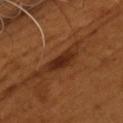{
  "biopsy_status": "not biopsied; imaged during a skin examination",
  "site": "chest",
  "patient": {
    "sex": "male",
    "age_approx": 55
  },
  "image": {
    "source": "total-body photography crop",
    "field_of_view_mm": 15
  },
  "lesion_size": {
    "long_diameter_mm_approx": 3.5
  },
  "automated_metrics": {
    "vs_skin_darker_L": 10.0,
    "vs_skin_contrast_norm": 10.0,
    "border_irregularity_0_10": 3.5,
    "color_variation_0_10": 2.0,
    "peripheral_color_asymmetry": 0.5
  }
}